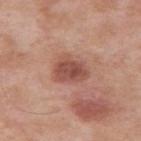biopsy status: catalogued during a skin exam; not biopsied.